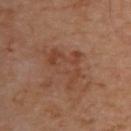Assessment:
Captured during whole-body skin photography for melanoma surveillance; the lesion was not biopsied.
Background:
Captured under cross-polarized illumination. The lesion is on the upper back. Cropped from a whole-body photographic skin survey; the tile spans about 15 mm. About 6 mm across. The subject is a male roughly 65 years of age.